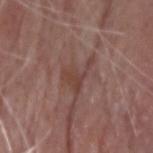<lesion>
<image>
  <source>total-body photography crop</source>
  <field_of_view_mm>15</field_of_view_mm>
</image>
<patient>
  <sex>male</sex>
  <age_approx>80</age_approx>
</patient>
<lighting>white-light</lighting>
<site>head or neck</site>
<lesion_size>
  <long_diameter_mm_approx>4.5</long_diameter_mm_approx>
</lesion_size>
</lesion>